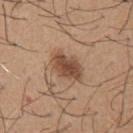follow-up = imaged on a skin check; not biopsied
lighting = white-light
acquisition = ~15 mm crop, total-body skin-cancer survey
patient = male, in their mid-60s
location = the chest